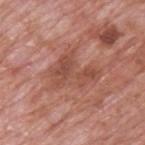Acquisition and patient details: A male patient, aged around 70. From the upper back. The lesion's longest dimension is about 5.5 mm. A lesion tile, about 15 mm wide, cut from a 3D total-body photograph.